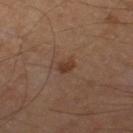Assessment: The lesion was photographed on a routine skin check and not biopsied; there is no pathology result. Clinical summary: Approximately 2 mm at its widest. The lesion is on the left thigh. The total-body-photography lesion software estimated border irregularity of about 3 on a 0–10 scale, a within-lesion color-variation index near 1.5/10, and radial color variation of about 0.5. A male subject aged 63 to 67. A close-up tile cropped from a whole-body skin photograph, about 15 mm across. Imaged with cross-polarized lighting.